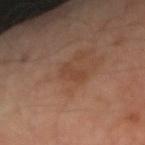This lesion was catalogued during total-body skin photography and was not selected for biopsy.
An algorithmic analysis of the crop reported a footprint of about 3.5 mm² and a shape-asymmetry score of about 0.35 (0 = symmetric). The software also gave an average lesion color of about L≈41 a*≈20 b*≈29 (CIELAB), roughly 6 lightness units darker than nearby skin, and a normalized border contrast of about 5. And it measured a nevus-likeness score of about 0/100 and a lesion-detection confidence of about 100/100.
Located on the left arm.
Approximately 3 mm at its widest.
A male patient aged 48 to 52.
Cropped from a total-body skin-imaging series; the visible field is about 15 mm.
This is a cross-polarized tile.